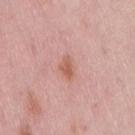Imaged during a routine full-body skin examination; the lesion was not biopsied and no histopathology is available. Located on the lower back. A close-up tile cropped from a whole-body skin photograph, about 15 mm across. A female patient, aged 48 to 52.